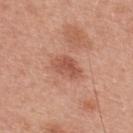Impression: Recorded during total-body skin imaging; not selected for excision or biopsy. Acquisition and patient details: The tile uses white-light illumination. The lesion's longest dimension is about 4 mm. An algorithmic analysis of the crop reported a classifier nevus-likeness of about 20/100. A 15 mm close-up extracted from a 3D total-body photography capture. A male subject, roughly 30 years of age. From the upper back.